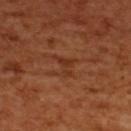Q: Was this lesion biopsied?
A: total-body-photography surveillance lesion; no biopsy
Q: What are the patient's age and sex?
A: female, about 55 years old
Q: What is the lesion's diameter?
A: ≈3 mm
Q: How was this image acquired?
A: ~15 mm tile from a whole-body skin photo
Q: Lesion location?
A: the upper back
Q: Illumination type?
A: cross-polarized